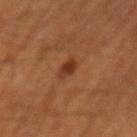Impression:
Recorded during total-body skin imaging; not selected for excision or biopsy.
Clinical summary:
On the mid back. A region of skin cropped from a whole-body photographic capture, roughly 15 mm wide. An algorithmic analysis of the crop reported an eccentricity of roughly 0.7 and a shape-asymmetry score of about 0.25 (0 = symmetric). The software also gave a lesion color around L≈31 a*≈22 b*≈30 in CIELAB and about 9 CIELAB-L* units darker than the surrounding skin. It also reported border irregularity of about 2 on a 0–10 scale and a peripheral color-asymmetry measure near 1. The software also gave a classifier nevus-likeness of about 95/100 and a lesion-detection confidence of about 100/100. Captured under cross-polarized illumination. A male subject, about 65 years old. The recorded lesion diameter is about 2.5 mm.